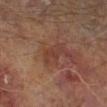Q: Was this lesion biopsied?
A: no biopsy performed (imaged during a skin exam)
Q: What is the imaging modality?
A: total-body-photography crop, ~15 mm field of view
Q: Where on the body is the lesion?
A: the right lower leg
Q: Who is the patient?
A: male, aged around 65
Q: What lighting was used for the tile?
A: cross-polarized illumination
Q: What is the lesion's diameter?
A: ≈3.5 mm